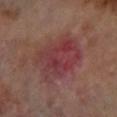No biopsy was performed on this lesion — it was imaged during a full skin examination and was not determined to be concerning. The lesion's longest dimension is about 6 mm. A male patient about 70 years old. A 15 mm crop from a total-body photograph taken for skin-cancer surveillance. From the left lower leg.